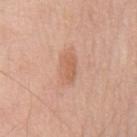  biopsy_status: not biopsied; imaged during a skin examination
  patient:
    sex: male
    age_approx: 70
  site: chest
  image:
    source: total-body photography crop
    field_of_view_mm: 15